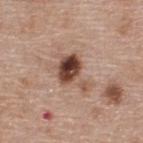• notes — no biopsy performed (imaged during a skin exam)
• lesion size — about 5 mm
• acquisition — ~15 mm crop, total-body skin-cancer survey
• patient — male, in their mid- to late 50s
• illumination — white-light illumination